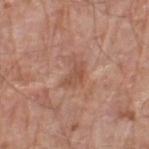Captured during whole-body skin photography for melanoma surveillance; the lesion was not biopsied. On the leg. A male subject, approximately 80 years of age. A lesion tile, about 15 mm wide, cut from a 3D total-body photograph.